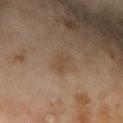The lesion was photographed on a routine skin check and not biopsied; there is no pathology result. Imaged with cross-polarized lighting. A close-up tile cropped from a whole-body skin photograph, about 15 mm across. A female subject aged 58–62. The lesion's longest dimension is about 3 mm. Automated image analysis of the tile measured an area of roughly 4.5 mm² and two-axis asymmetry of about 0.3. Located on the right forearm.